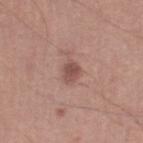Captured during whole-body skin photography for melanoma surveillance; the lesion was not biopsied.
This image is a 15 mm lesion crop taken from a total-body photograph.
Captured under white-light illumination.
On the right lower leg.
The lesion's longest dimension is about 2.5 mm.
A male patient, in their mid-40s.